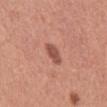Case summary:
* biopsy status · imaged on a skin check; not biopsied
* body site · the front of the torso
* image-analysis metrics · a lesion color around L≈51 a*≈26 b*≈28 in CIELAB and a lesion–skin lightness drop of about 12; a border-irregularity rating of about 2.5/10, internal color variation of about 2 on a 0–10 scale, and a peripheral color-asymmetry measure near 0.5; a nevus-likeness score of about 80/100 and a lesion-detection confidence of about 100/100
* acquisition · ~15 mm tile from a whole-body skin photo
* patient · male, about 45 years old